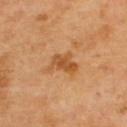{"biopsy_status": "not biopsied; imaged during a skin examination", "image": {"source": "total-body photography crop", "field_of_view_mm": 15}, "lesion_size": {"long_diameter_mm_approx": 3.5}, "site": "upper back", "patient": {"sex": "male", "age_approx": 70}}